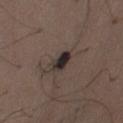Impression:
The lesion was photographed on a routine skin check and not biopsied; there is no pathology result.
Clinical summary:
From the abdomen. This is a white-light tile. A roughly 15 mm field-of-view crop from a total-body skin photograph. A male subject, aged approximately 50. Automated tile analysis of the lesion measured a footprint of about 4.5 mm². The analysis additionally found an average lesion color of about L≈28 a*≈9 b*≈14 (CIELAB). The analysis additionally found border irregularity of about 2.5 on a 0–10 scale, internal color variation of about 5.5 on a 0–10 scale, and radial color variation of about 1.5. And it measured a nevus-likeness score of about 10/100 and a detector confidence of about 100 out of 100 that the crop contains a lesion. Longest diameter approximately 3 mm.